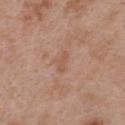biopsy status — total-body-photography surveillance lesion; no biopsy | anatomic site — the upper back | size — about 2.5 mm | acquisition — total-body-photography crop, ~15 mm field of view | patient — female, aged 28 to 32.